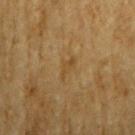The lesion was tiled from a total-body skin photograph and was not biopsied.
The patient is a male roughly 85 years of age or older.
This image is a 15 mm lesion crop taken from a total-body photograph.
On the right upper arm.
Longest diameter approximately 3 mm.